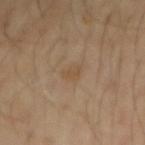Context: Approximately 2.5 mm at its widest. The total-body-photography lesion software estimated an automated nevus-likeness rating near 0 out of 100 and a lesion-detection confidence of about 100/100. A male subject, about 45 years old. A lesion tile, about 15 mm wide, cut from a 3D total-body photograph. The lesion is on the right forearm.